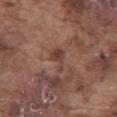| feature | finding |
|---|---|
| biopsy status | total-body-photography surveillance lesion; no biopsy |
| diameter | about 3.5 mm |
| automated metrics | a lesion area of about 4.5 mm² and a symmetry-axis asymmetry near 0.5; a mean CIELAB color near L≈41 a*≈19 b*≈25, roughly 8 lightness units darker than nearby skin, and a normalized border contrast of about 6.5; border irregularity of about 5 on a 0–10 scale and a within-lesion color-variation index near 2.5/10 |
| location | the abdomen |
| lighting | white-light |
| patient | male, approximately 75 years of age |
| image source | ~15 mm tile from a whole-body skin photo |